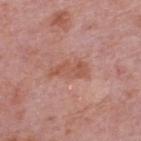The lesion was tiled from a total-body skin photograph and was not biopsied. Approximately 5 mm at its widest. A male subject aged around 75. From the upper back. A 15 mm close-up extracted from a 3D total-body photography capture.